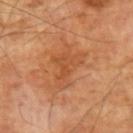notes — imaged on a skin check; not biopsied
illumination — cross-polarized illumination
image — ~15 mm tile from a whole-body skin photo
diameter — ~5 mm (longest diameter)
patient — male, aged approximately 70
body site — the left upper arm